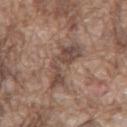Recorded during total-body skin imaging; not selected for excision or biopsy. Located on the chest. This is a white-light tile. Approximately 7 mm at its widest. A roughly 15 mm field-of-view crop from a total-body skin photograph. A male subject, aged around 75.